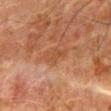Captured during whole-body skin photography for melanoma surveillance; the lesion was not biopsied.
On the abdomen.
Automated image analysis of the tile measured a border-irregularity rating of about 3/10, a color-variation rating of about 1.5/10, and peripheral color asymmetry of about 0.5.
A lesion tile, about 15 mm wide, cut from a 3D total-body photograph.
Imaged with cross-polarized lighting.
A male subject in their 80s.